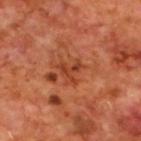{
  "biopsy_status": "not biopsied; imaged during a skin examination",
  "automated_metrics": {
    "eccentricity": 0.45,
    "shape_asymmetry": 0.65,
    "lesion_detection_confidence_0_100": 100
  },
  "site": "upper back",
  "lighting": "cross-polarized",
  "lesion_size": {
    "long_diameter_mm_approx": 3.0
  },
  "image": {
    "source": "total-body photography crop",
    "field_of_view_mm": 15
  },
  "patient": {
    "sex": "male",
    "age_approx": 70
  }
}